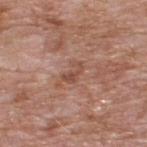biopsy status — imaged on a skin check; not biopsied
site — the upper back
patient — male, aged approximately 60
image — total-body-photography crop, ~15 mm field of view
lighting — white-light illumination
lesion size — about 2.5 mm